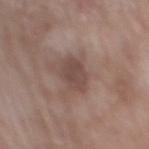Impression: The lesion was photographed on a routine skin check and not biopsied; there is no pathology result. Image and clinical context: A 15 mm close-up extracted from a 3D total-body photography capture. Located on the mid back. A male subject, aged 63 to 67. Approximately 3 mm at its widest.